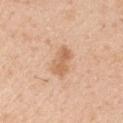{
  "biopsy_status": "not biopsied; imaged during a skin examination",
  "patient": {
    "sex": "male",
    "age_approx": 40
  },
  "image": {
    "source": "total-body photography crop",
    "field_of_view_mm": 15
  },
  "site": "right upper arm"
}